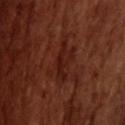{"biopsy_status": "not biopsied; imaged during a skin examination", "patient": {"sex": "male", "age_approx": 70}, "site": "head or neck", "automated_metrics": {"area_mm2_approx": 11.0, "eccentricity": 0.85, "border_irregularity_0_10": 6.0, "peripheral_color_asymmetry": 1.0}, "lesion_size": {"long_diameter_mm_approx": 5.5}, "lighting": "cross-polarized", "image": {"source": "total-body photography crop", "field_of_view_mm": 15}}